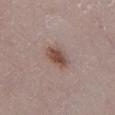patient: male, approximately 80 years of age
lighting: white-light
image-analysis metrics: an eccentricity of roughly 0.7 and a shape-asymmetry score of about 0.25 (0 = symmetric); a mean CIELAB color near L≈49 a*≈18 b*≈24, roughly 11 lightness units darker than nearby skin, and a lesion-to-skin contrast of about 9 (normalized; higher = more distinct); a nevus-likeness score of about 95/100 and a lesion-detection confidence of about 100/100
lesion size: about 3.5 mm
acquisition: 15 mm crop, total-body photography
anatomic site: the left lower leg